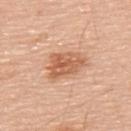follow-up: catalogued during a skin exam; not biopsied | lighting: white-light | image source: ~15 mm tile from a whole-body skin photo | patient: male, aged approximately 70 | size: ~5 mm (longest diameter) | location: the upper back.